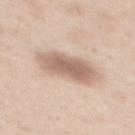Recorded during total-body skin imaging; not selected for excision or biopsy.
The lesion-visualizer software estimated a lesion color around L≈63 a*≈17 b*≈27 in CIELAB and a normalized border contrast of about 8. The software also gave a border-irregularity rating of about 2/10.
A lesion tile, about 15 mm wide, cut from a 3D total-body photograph.
Imaged with white-light lighting.
A female subject, aged around 50.
From the mid back.